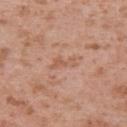Imaged during a routine full-body skin examination; the lesion was not biopsied and no histopathology is available. From the left upper arm. A male patient, aged 38–42. A region of skin cropped from a whole-body photographic capture, roughly 15 mm wide. About 3 mm across. Captured under white-light illumination.